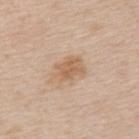illumination: white-light
lesion diameter: about 4 mm
image-analysis metrics: an area of roughly 8.5 mm², an eccentricity of roughly 0.75, and two-axis asymmetry of about 0.3; a border-irregularity rating of about 3.5/10, a within-lesion color-variation index near 2.5/10, and radial color variation of about 1; a classifier nevus-likeness of about 20/100 and a lesion-detection confidence of about 100/100
anatomic site: the upper back
image source: total-body-photography crop, ~15 mm field of view
patient: male, in their mid-60s